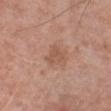notes=total-body-photography surveillance lesion; no biopsy
lighting=white-light
body site=the right lower leg
image source=15 mm crop, total-body photography
patient=male, roughly 80 years of age
TBP lesion metrics=border irregularity of about 3 on a 0–10 scale, a color-variation rating of about 1.5/10, and radial color variation of about 0.5